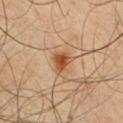* biopsy status — total-body-photography surveillance lesion; no biopsy
* TBP lesion metrics — a color-variation rating of about 4/10 and a peripheral color-asymmetry measure near 1; a nevus-likeness score of about 95/100
* image source — ~15 mm crop, total-body skin-cancer survey
* body site — the chest
* lesion diameter — ~3 mm (longest diameter)
* tile lighting — cross-polarized
* subject — male, aged 38 to 42